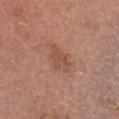Q: Was a biopsy performed?
A: imaged on a skin check; not biopsied
Q: Lesion location?
A: the head or neck
Q: Lesion size?
A: ≈3.5 mm
Q: Illumination type?
A: white-light
Q: What are the patient's age and sex?
A: male, aged 58 to 62
Q: How was this image acquired?
A: total-body-photography crop, ~15 mm field of view